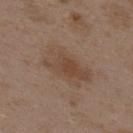The lesion was tiled from a total-body skin photograph and was not biopsied. Longest diameter approximately 6 mm. The patient is a female aged around 35. Cropped from a whole-body photographic skin survey; the tile spans about 15 mm. The lesion is located on the upper back. An algorithmic analysis of the crop reported a lesion area of about 18 mm², an eccentricity of roughly 0.85, and a symmetry-axis asymmetry near 0.2. The software also gave border irregularity of about 2.5 on a 0–10 scale and peripheral color asymmetry of about 1. And it measured a nevus-likeness score of about 10/100 and a detector confidence of about 100 out of 100 that the crop contains a lesion.